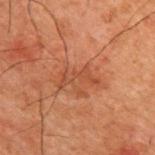  biopsy_status: not biopsied; imaged during a skin examination
  patient:
    sex: male
    age_approx: 50
  site: upper back
  image:
    source: total-body photography crop
    field_of_view_mm: 15